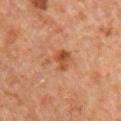Impression: This lesion was catalogued during total-body skin photography and was not selected for biopsy. Context: A male patient roughly 60 years of age. The lesion is on the chest. Cropped from a whole-body photographic skin survey; the tile spans about 15 mm. Longest diameter approximately 4 mm.